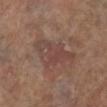No biopsy was performed on this lesion — it was imaged during a full skin examination and was not determined to be concerning. From the right lower leg. The subject is a female approximately 75 years of age. This image is a 15 mm lesion crop taken from a total-body photograph. This is a cross-polarized tile.